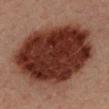follow-up: imaged on a skin check; not biopsied | lesion diameter: ~11 mm (longest diameter) | acquisition: total-body-photography crop, ~15 mm field of view | anatomic site: the back | subject: male, in their 30s | tile lighting: cross-polarized.